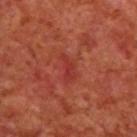{"image": {"source": "total-body photography crop", "field_of_view_mm": 15}, "patient": {"sex": "male", "age_approx": 70}, "site": "upper back"}